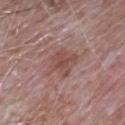The lesion was tiled from a total-body skin photograph and was not biopsied.
A male subject, aged approximately 65.
Imaged with white-light lighting.
This image is a 15 mm lesion crop taken from a total-body photograph.
The lesion-visualizer software estimated an area of roughly 9.5 mm², an eccentricity of roughly 0.7, and two-axis asymmetry of about 0.3. It also reported an average lesion color of about L≈49 a*≈21 b*≈24 (CIELAB), about 8 CIELAB-L* units darker than the surrounding skin, and a normalized border contrast of about 6.5. The software also gave a border-irregularity index near 4/10 and radial color variation of about 1.
The lesion is located on the chest.